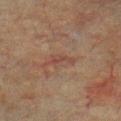Part of a total-body skin-imaging series; this lesion was reviewed on a skin check and was not flagged for biopsy. A region of skin cropped from a whole-body photographic capture, roughly 15 mm wide. The lesion is located on the chest. About 3 mm across. The subject is a male in their mid- to late 70s. Automated image analysis of the tile measured an area of roughly 2 mm², an eccentricity of roughly 0.95, and two-axis asymmetry of about 0.5. The analysis additionally found an average lesion color of about L≈36 a*≈18 b*≈22 (CIELAB), a lesion–skin lightness drop of about 6, and a lesion-to-skin contrast of about 5.5 (normalized; higher = more distinct). And it measured border irregularity of about 6 on a 0–10 scale, internal color variation of about 0 on a 0–10 scale, and peripheral color asymmetry of about 0.